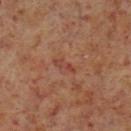{"lesion_size": {"long_diameter_mm_approx": 3.0}, "patient": {"sex": "male", "age_approx": 60}, "image": {"source": "total-body photography crop", "field_of_view_mm": 15}, "lighting": "cross-polarized", "site": "left lower leg"}